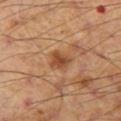This lesion was catalogued during total-body skin photography and was not selected for biopsy.
A male subject roughly 55 years of age.
Imaged with cross-polarized lighting.
On the left thigh.
Cropped from a whole-body photographic skin survey; the tile spans about 15 mm.